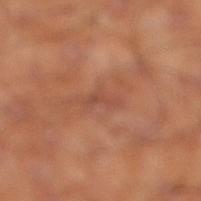Findings:
– biopsy status · imaged on a skin check; not biopsied
– site · the right lower leg
– lighting · cross-polarized
– subject · male, aged 58 to 62
– image source · ~15 mm crop, total-body skin-cancer survey